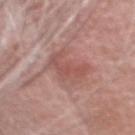No biopsy was performed on this lesion — it was imaged during a full skin examination and was not determined to be concerning. A 15 mm crop from a total-body photograph taken for skin-cancer surveillance. Approximately 4 mm at its widest. This is a white-light tile. The subject is a male aged approximately 65. On the head or neck.